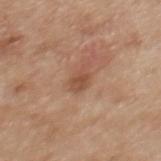Notes:
* notes · total-body-photography surveillance lesion; no biopsy
* subject · female, aged approximately 40
* illumination · white-light
* automated lesion analysis · an eccentricity of roughly 0.65 and a shape-asymmetry score of about 0.35 (0 = symmetric); an average lesion color of about L≈50 a*≈22 b*≈31 (CIELAB) and a lesion-to-skin contrast of about 6.5 (normalized; higher = more distinct); a border-irregularity index near 3.5/10 and a within-lesion color-variation index near 2/10; a classifier nevus-likeness of about 0/100 and a detector confidence of about 100 out of 100 that the crop contains a lesion
* body site · the mid back
* imaging modality · ~15 mm crop, total-body skin-cancer survey
* size · ≈2.5 mm